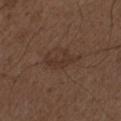Impression: Recorded during total-body skin imaging; not selected for excision or biopsy. Clinical summary: The patient is a male aged 48 to 52. A roughly 15 mm field-of-view crop from a total-body skin photograph. On the left upper arm.